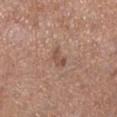Part of a total-body skin-imaging series; this lesion was reviewed on a skin check and was not flagged for biopsy. The lesion is on the left lower leg. Captured under white-light illumination. A 15 mm close-up extracted from a 3D total-body photography capture. Longest diameter approximately 2.5 mm. Automated image analysis of the tile measured border irregularity of about 3 on a 0–10 scale, internal color variation of about 2.5 on a 0–10 scale, and radial color variation of about 1. A female subject, aged approximately 60.